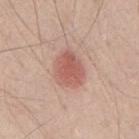{"biopsy_status": "not biopsied; imaged during a skin examination", "lesion_size": {"long_diameter_mm_approx": 4.0}, "site": "mid back", "lighting": "white-light", "image": {"source": "total-body photography crop", "field_of_view_mm": 15}, "patient": {"sex": "male", "age_approx": 50}}